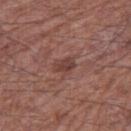{"biopsy_status": "not biopsied; imaged during a skin examination", "patient": {"sex": "male", "age_approx": 65}, "image": {"source": "total-body photography crop", "field_of_view_mm": 15}, "lighting": "white-light", "site": "right thigh", "lesion_size": {"long_diameter_mm_approx": 2.5}}